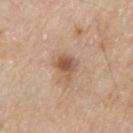Impression:
Captured during whole-body skin photography for melanoma surveillance; the lesion was not biopsied.
Image and clinical context:
Measured at roughly 3 mm in maximum diameter. On the right upper arm. A male subject aged around 80. An algorithmic analysis of the crop reported an area of roughly 5.5 mm², a shape eccentricity near 0.55, and two-axis asymmetry of about 0.3. The analysis additionally found a border-irregularity index near 3.5/10 and radial color variation of about 1.5. This image is a 15 mm lesion crop taken from a total-body photograph.